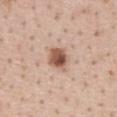Part of a total-body skin-imaging series; this lesion was reviewed on a skin check and was not flagged for biopsy. The lesion's longest dimension is about 3 mm. A female subject approximately 45 years of age. Cropped from a total-body skin-imaging series; the visible field is about 15 mm. An algorithmic analysis of the crop reported an average lesion color of about L≈54 a*≈21 b*≈29 (CIELAB), about 16 CIELAB-L* units darker than the surrounding skin, and a normalized lesion–skin contrast near 10.5. It also reported border irregularity of about 2 on a 0–10 scale, a color-variation rating of about 6.5/10, and peripheral color asymmetry of about 2.5. It also reported a lesion-detection confidence of about 100/100. Captured under white-light illumination. From the front of the torso.